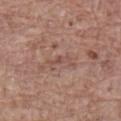acquisition: ~15 mm tile from a whole-body skin photo
patient: male, aged 78 to 82
lighting: white-light
site: the lower back